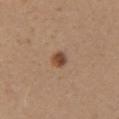workup: no biopsy performed (imaged during a skin exam).